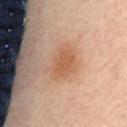Part of a total-body skin-imaging series; this lesion was reviewed on a skin check and was not flagged for biopsy.
Located on the back.
The lesion's longest dimension is about 4.5 mm.
The subject is a male about 85 years old or older.
Automated tile analysis of the lesion measured a mean CIELAB color near L≈55 a*≈21 b*≈33 and about 8 CIELAB-L* units darker than the surrounding skin. The software also gave a border-irregularity index near 2.5/10 and radial color variation of about 1.
This is a cross-polarized tile.
Cropped from a total-body skin-imaging series; the visible field is about 15 mm.